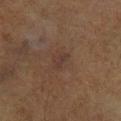| key | value |
|---|---|
| workup | catalogued during a skin exam; not biopsied |